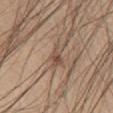biopsy_status: not biopsied; imaged during a skin examination
image:
  source: total-body photography crop
  field_of_view_mm: 15
site: chest
lesion_size:
  long_diameter_mm_approx: 3.5
patient:
  sex: male
  age_approx: 65